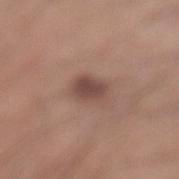Imaged during a routine full-body skin examination; the lesion was not biopsied and no histopathology is available. A 15 mm close-up tile from a total-body photography series done for melanoma screening. From the left lower leg. The lesion's longest dimension is about 3 mm. A male subject, aged 28 to 32. This is a white-light tile.